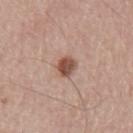Captured during whole-body skin photography for melanoma surveillance; the lesion was not biopsied. A region of skin cropped from a whole-body photographic capture, roughly 15 mm wide. The lesion is located on the back. A male patient, aged around 65.